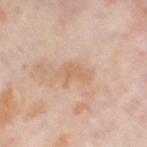Part of a total-body skin-imaging series; this lesion was reviewed on a skin check and was not flagged for biopsy. This is a cross-polarized tile. A female subject, roughly 55 years of age. The total-body-photography lesion software estimated a lesion color around L≈64 a*≈19 b*≈33 in CIELAB, about 7 CIELAB-L* units darker than the surrounding skin, and a normalized lesion–skin contrast near 5.5. And it measured a classifier nevus-likeness of about 0/100 and lesion-presence confidence of about 100/100. A region of skin cropped from a whole-body photographic capture, roughly 15 mm wide. From the right thigh.